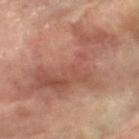Acquisition and patient details: The lesion is located on the arm. A 15 mm close-up tile from a total-body photography series done for melanoma screening. A female patient, about 75 years old.